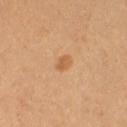Imaged during a routine full-body skin examination; the lesion was not biopsied and no histopathology is available.
A roughly 15 mm field-of-view crop from a total-body skin photograph.
A female patient aged 53–57.
The lesion is located on the right thigh.